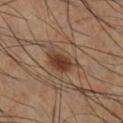Q: Was this lesion biopsied?
A: total-body-photography surveillance lesion; no biopsy
Q: What are the patient's age and sex?
A: male, in their 60s
Q: What lighting was used for the tile?
A: cross-polarized illumination
Q: Automated lesion metrics?
A: a lesion color around L≈38 a*≈18 b*≈27 in CIELAB, a lesion–skin lightness drop of about 10, and a normalized lesion–skin contrast near 9
Q: How large is the lesion?
A: about 4.5 mm
Q: Lesion location?
A: the right lower leg
Q: What kind of image is this?
A: ~15 mm tile from a whole-body skin photo